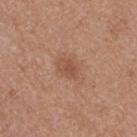biopsy status: total-body-photography surveillance lesion; no biopsy
image-analysis metrics: an eccentricity of roughly 0.65 and a symmetry-axis asymmetry near 0.25; roughly 8 lightness units darker than nearby skin and a normalized border contrast of about 5.5
lesion size: ≈3 mm
patient: female, aged around 40
location: the right thigh
image source: total-body-photography crop, ~15 mm field of view